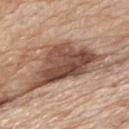• image: ~15 mm crop, total-body skin-cancer survey
• tile lighting: white-light
• image-analysis metrics: lesion-presence confidence of about 95/100
• subject: male, aged approximately 80
• size: ~8 mm (longest diameter)
• anatomic site: the upper back